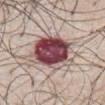Impression:
This lesion was catalogued during total-body skin photography and was not selected for biopsy.
Clinical summary:
A 15 mm close-up tile from a total-body photography series done for melanoma screening. A male patient about 70 years old. Automated tile analysis of the lesion measured a mean CIELAB color near L≈48 a*≈23 b*≈18, roughly 21 lightness units darker than nearby skin, and a normalized lesion–skin contrast near 14.5. It also reported peripheral color asymmetry of about 4.5. The software also gave an automated nevus-likeness rating near 10 out of 100 and lesion-presence confidence of about 100/100. The recorded lesion diameter is about 7 mm. This is a white-light tile. Located on the chest.